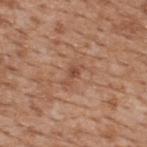The lesion was photographed on a routine skin check and not biopsied; there is no pathology result.
The lesion is located on the upper back.
A lesion tile, about 15 mm wide, cut from a 3D total-body photograph.
Longest diameter approximately 2.5 mm.
A male patient approximately 65 years of age.
This is a white-light tile.
The lesion-visualizer software estimated a lesion color around L≈49 a*≈23 b*≈31 in CIELAB, roughly 8 lightness units darker than nearby skin, and a lesion-to-skin contrast of about 6 (normalized; higher = more distinct).